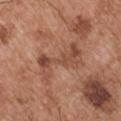Q: Is there a histopathology result?
A: catalogued during a skin exam; not biopsied
Q: How large is the lesion?
A: ≈6 mm
Q: How was the tile lit?
A: white-light illumination
Q: What did automated image analysis measure?
A: an area of roughly 11 mm², an outline eccentricity of about 0.9 (0 = round, 1 = elongated), and a symmetry-axis asymmetry near 0.35; a border-irregularity index near 7.5/10, a within-lesion color-variation index near 4.5/10, and a peripheral color-asymmetry measure near 1; an automated nevus-likeness rating near 0 out of 100 and a detector confidence of about 95 out of 100 that the crop contains a lesion
Q: Patient demographics?
A: male, in their mid- to late 50s
Q: Where on the body is the lesion?
A: the left upper arm
Q: What is the imaging modality?
A: total-body-photography crop, ~15 mm field of view